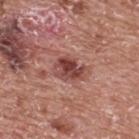This lesion was catalogued during total-body skin photography and was not selected for biopsy. A close-up tile cropped from a whole-body skin photograph, about 15 mm across. This is a white-light tile. The lesion is on the upper back. About 3.5 mm across. A male patient, in their 70s. The lesion-visualizer software estimated a lesion color around L≈44 a*≈26 b*≈24 in CIELAB, about 13 CIELAB-L* units darker than the surrounding skin, and a lesion-to-skin contrast of about 10 (normalized; higher = more distinct).